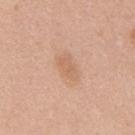The lesion was photographed on a routine skin check and not biopsied; there is no pathology result. A 15 mm close-up extracted from a 3D total-body photography capture. The subject is a male in their mid- to late 40s. This is a white-light tile. Located on the mid back.